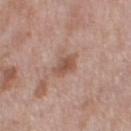Q: How was this image acquired?
A: ~15 mm crop, total-body skin-cancer survey
Q: Lesion location?
A: the right thigh
Q: Illumination type?
A: white-light
Q: Lesion size?
A: ≈3 mm
Q: Patient demographics?
A: female, approximately 50 years of age